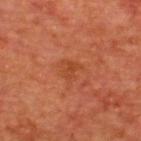The lesion was photographed on a routine skin check and not biopsied; there is no pathology result.